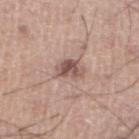Imaged during a routine full-body skin examination; the lesion was not biopsied and no histopathology is available. The recorded lesion diameter is about 3.5 mm. A 15 mm close-up extracted from a 3D total-body photography capture. A male subject aged approximately 20. Located on the left lower leg. Captured under white-light illumination.